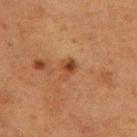{
  "biopsy_status": "not biopsied; imaged during a skin examination",
  "image": {
    "source": "total-body photography crop",
    "field_of_view_mm": 15
  },
  "site": "left thigh",
  "patient": {
    "sex": "female",
    "age_approx": 50
  },
  "lighting": "cross-polarized",
  "lesion_size": {
    "long_diameter_mm_approx": 2.5
  }
}